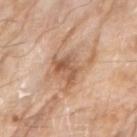The lesion was photographed on a routine skin check and not biopsied; there is no pathology result. A roughly 15 mm field-of-view crop from a total-body skin photograph. The tile uses white-light illumination. On the arm. A male subject aged 78–82.